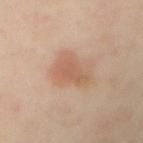Imaged during a routine full-body skin examination; the lesion was not biopsied and no histopathology is available.
About 4.5 mm across.
A male patient, roughly 50 years of age.
Captured under cross-polarized illumination.
Cropped from a total-body skin-imaging series; the visible field is about 15 mm.
The total-body-photography lesion software estimated a border-irregularity rating of about 4/10 and peripheral color asymmetry of about 1.
The lesion is on the left arm.